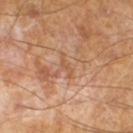notes = no biopsy performed (imaged during a skin exam); lesion diameter = ≈3 mm; subject = male, about 65 years old; image = total-body-photography crop, ~15 mm field of view; illumination = cross-polarized.